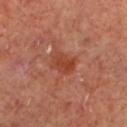Impression:
Recorded during total-body skin imaging; not selected for excision or biopsy.
Clinical summary:
A male subject, aged around 65. A 15 mm crop from a total-body photograph taken for skin-cancer surveillance. Located on the left lower leg.